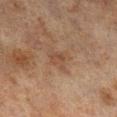Impression: No biopsy was performed on this lesion — it was imaged during a full skin examination and was not determined to be concerning. Clinical summary: A male patient about 70 years old. The tile uses cross-polarized illumination. Longest diameter approximately 3 mm. This image is a 15 mm lesion crop taken from a total-body photograph. The lesion-visualizer software estimated roughly 5 lightness units darker than nearby skin. The software also gave a nevus-likeness score of about 0/100. On the right lower leg.